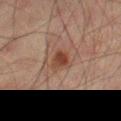Q: Is there a histopathology result?
A: catalogued during a skin exam; not biopsied
Q: Patient demographics?
A: male, in their 50s
Q: What is the imaging modality?
A: 15 mm crop, total-body photography
Q: Where on the body is the lesion?
A: the right thigh